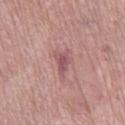Clinical impression: Captured during whole-body skin photography for melanoma surveillance; the lesion was not biopsied. Acquisition and patient details: The lesion-visualizer software estimated an area of roughly 4 mm², an eccentricity of roughly 0.85, and a shape-asymmetry score of about 0.4 (0 = symmetric). And it measured a border-irregularity rating of about 4/10 and internal color variation of about 2 on a 0–10 scale. The software also gave a classifier nevus-likeness of about 0/100. Measured at roughly 3 mm in maximum diameter. A male patient aged 58–62. The lesion is on the mid back. This is a white-light tile. A 15 mm crop from a total-body photograph taken for skin-cancer surveillance.